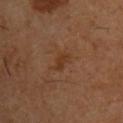follow-up: no biopsy performed (imaged during a skin exam)
patient: male, in their mid- to late 50s
imaging modality: 15 mm crop, total-body photography
body site: the upper back